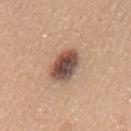Part of a total-body skin-imaging series; this lesion was reviewed on a skin check and was not flagged for biopsy. Cropped from a total-body skin-imaging series; the visible field is about 15 mm. This is a white-light tile. Measured at roughly 4.5 mm in maximum diameter. The patient is a female about 55 years old. Automated image analysis of the tile measured a lesion–skin lightness drop of about 18 and a normalized lesion–skin contrast near 12.5. And it measured border irregularity of about 2 on a 0–10 scale, internal color variation of about 8.5 on a 0–10 scale, and a peripheral color-asymmetry measure near 2.5. From the mid back.